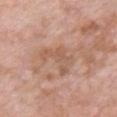biopsy status — catalogued during a skin exam; not biopsied
lighting — white-light
diameter — ≈4.5 mm
location — the chest
patient — male, aged approximately 50
automated lesion analysis — a footprint of about 9 mm², an outline eccentricity of about 0.8 (0 = round, 1 = elongated), and a shape-asymmetry score of about 0.6 (0 = symmetric); a mean CIELAB color near L≈57 a*≈20 b*≈30, about 7 CIELAB-L* units darker than the surrounding skin, and a normalized border contrast of about 5; a border-irregularity index near 7/10, internal color variation of about 3 on a 0–10 scale, and peripheral color asymmetry of about 1; an automated nevus-likeness rating near 0 out of 100
acquisition — total-body-photography crop, ~15 mm field of view